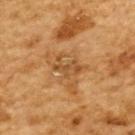workup — imaged on a skin check; not biopsied
imaging modality — 15 mm crop, total-body photography
site — the upper back
subject — male, aged 83 or over